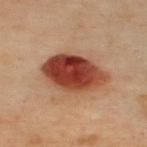{
  "patient": {
    "sex": "male",
    "age_approx": 50
  },
  "automated_metrics": {
    "cielab_L": 34,
    "cielab_a": 25,
    "cielab_b": 27,
    "vs_skin_darker_L": 16.0,
    "vs_skin_contrast_norm": 14.0,
    "border_irregularity_0_10": 2.0,
    "color_variation_0_10": 6.5,
    "peripheral_color_asymmetry": 2.0,
    "nevus_likeness_0_100": 100,
    "lesion_detection_confidence_0_100": 100
  },
  "site": "chest",
  "lesion_size": {
    "long_diameter_mm_approx": 7.5
  },
  "image": {
    "source": "total-body photography crop",
    "field_of_view_mm": 15
  }
}